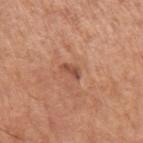Findings:
- biopsy status · no biopsy performed (imaged during a skin exam)
- lesion diameter · ~2.5 mm (longest diameter)
- automated metrics · a lesion area of about 3 mm², an outline eccentricity of about 0.85 (0 = round, 1 = elongated), and a symmetry-axis asymmetry near 0.3; a lesion color around L≈51 a*≈24 b*≈31 in CIELAB, roughly 10 lightness units darker than nearby skin, and a normalized border contrast of about 7
- lighting · white-light illumination
- patient · male, aged approximately 65
- location · the left upper arm
- imaging modality · 15 mm crop, total-body photography